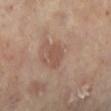Q: Was this lesion biopsied?
A: total-body-photography surveillance lesion; no biopsy
Q: What are the patient's age and sex?
A: female, in their 60s
Q: What lighting was used for the tile?
A: cross-polarized illumination
Q: Lesion size?
A: about 3 mm
Q: Where on the body is the lesion?
A: the left lower leg
Q: How was this image acquired?
A: total-body-photography crop, ~15 mm field of view